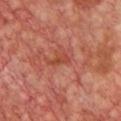No biopsy was performed on this lesion — it was imaged during a full skin examination and was not determined to be concerning. From the chest. A female subject aged 43 to 47. The lesion-visualizer software estimated a lesion area of about 2.5 mm² and an eccentricity of roughly 0.9. It also reported an average lesion color of about L≈45 a*≈30 b*≈32 (CIELAB), a lesion–skin lightness drop of about 7, and a normalized border contrast of about 6. And it measured lesion-presence confidence of about 95/100. Cropped from a whole-body photographic skin survey; the tile spans about 15 mm.